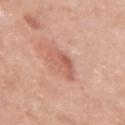{
  "site": "arm",
  "lesion_size": {
    "long_diameter_mm_approx": 4.0
  },
  "image": {
    "source": "total-body photography crop",
    "field_of_view_mm": 15
  },
  "patient": {
    "sex": "female",
    "age_approx": 40
  },
  "lighting": "white-light"
}